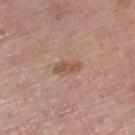| feature | finding |
|---|---|
| follow-up | imaged on a skin check; not biopsied |
| image | 15 mm crop, total-body photography |
| lighting | white-light |
| body site | the right lower leg |
| subject | male, approximately 70 years of age |